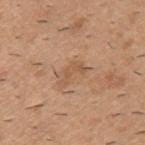workup = no biopsy performed (imaged during a skin exam) | illumination = white-light illumination | automated metrics = a border-irregularity rating of about 6.5/10, a color-variation rating of about 0.5/10, and radial color variation of about 0 | body site = the left upper arm | patient = male, aged around 40 | acquisition = ~15 mm crop, total-body skin-cancer survey.